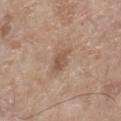biopsy_status: not biopsied; imaged during a skin examination
lighting: white-light
image:
  source: total-body photography crop
  field_of_view_mm: 15
site: right lower leg
automated_metrics:
  shape_asymmetry: 0.25
  cielab_L: 53
  cielab_a: 17
  cielab_b: 28
  vs_skin_darker_L: 9.0
  vs_skin_contrast_norm: 6.5
  nevus_likeness_0_100: 0
  lesion_detection_confidence_0_100: 100
patient:
  sex: male
  age_approx: 65
lesion_size:
  long_diameter_mm_approx: 3.0